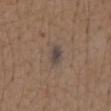workup: total-body-photography surveillance lesion; no biopsy | size: ~2.5 mm (longest diameter) | body site: the front of the torso | image-analysis metrics: an average lesion color of about L≈43 a*≈10 b*≈19 (CIELAB) and a lesion–skin lightness drop of about 8; a color-variation rating of about 3/10 and radial color variation of about 1; an automated nevus-likeness rating near 5 out of 100 and a detector confidence of about 100 out of 100 that the crop contains a lesion | patient: male, in their mid- to late 70s | image: total-body-photography crop, ~15 mm field of view | lighting: white-light.